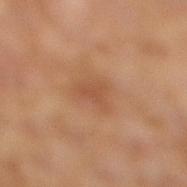Notes:
– notes · total-body-photography surveillance lesion; no biopsy
– location · the right lower leg
– patient · female, aged around 60
– image source · total-body-photography crop, ~15 mm field of view